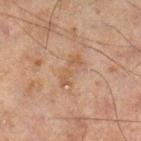workup = imaged on a skin check; not biopsied | patient = male, aged around 45 | acquisition = ~15 mm crop, total-body skin-cancer survey | lesion diameter = ≈4 mm | image-analysis metrics = a lesion–skin lightness drop of about 5 and a normalized lesion–skin contrast near 5.5; a border-irregularity index near 4.5/10, internal color variation of about 0.5 on a 0–10 scale, and radial color variation of about 0 | location = the right lower leg.